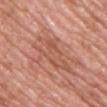Captured during whole-body skin photography for melanoma surveillance; the lesion was not biopsied. Located on the chest. The tile uses white-light illumination. Longest diameter approximately 7 mm. A male patient, about 50 years old. A lesion tile, about 15 mm wide, cut from a 3D total-body photograph.